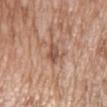Notes:
- follow-up: imaged on a skin check; not biopsied